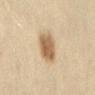Q: Was this lesion biopsied?
A: total-body-photography surveillance lesion; no biopsy
Q: Lesion location?
A: the back
Q: How large is the lesion?
A: ~3.5 mm (longest diameter)
Q: Patient demographics?
A: female, aged 58–62
Q: What lighting was used for the tile?
A: cross-polarized
Q: What did automated image analysis measure?
A: a lesion area of about 9.5 mm² and a symmetry-axis asymmetry near 0.15; an automated nevus-likeness rating near 100 out of 100 and a lesion-detection confidence of about 100/100
Q: What is the imaging modality?
A: total-body-photography crop, ~15 mm field of view